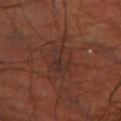notes: imaged on a skin check; not biopsied | location: the left thigh | image: ~15 mm crop, total-body skin-cancer survey | subject: male, aged 68 to 72.